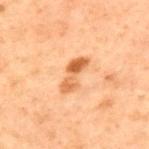Clinical impression: The lesion was photographed on a routine skin check and not biopsied; there is no pathology result. Context: A roughly 15 mm field-of-view crop from a total-body skin photograph. This is a cross-polarized tile. On the upper back. Automated image analysis of the tile measured an area of roughly 5.5 mm² and a shape-asymmetry score of about 0.35 (0 = symmetric). It also reported a lesion–skin lightness drop of about 11 and a normalized lesion–skin contrast near 8.5. The analysis additionally found a border-irregularity rating of about 5/10 and a within-lesion color-variation index near 4.5/10. The analysis additionally found a nevus-likeness score of about 85/100 and a detector confidence of about 100 out of 100 that the crop contains a lesion. A male subject, roughly 70 years of age.